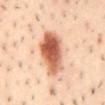workup — catalogued during a skin exam; not biopsied | anatomic site — the back | image source — 15 mm crop, total-body photography | patient — male, aged 38 to 42 | lighting — cross-polarized | lesion diameter — about 7.5 mm.